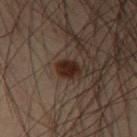No biopsy was performed on this lesion — it was imaged during a full skin examination and was not determined to be concerning. Automated image analysis of the tile measured a within-lesion color-variation index near 1.5/10 and a peripheral color-asymmetry measure near 0.5. The tile uses cross-polarized illumination. The lesion's longest dimension is about 2.5 mm. A male subject, aged around 55. A roughly 15 mm field-of-view crop from a total-body skin photograph. The lesion is located on the left thigh.